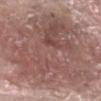  biopsy_status: not biopsied; imaged during a skin examination
  site: head or neck
  patient:
    sex: male
    age_approx: 65
  automated_metrics:
    eccentricity: 0.85
    shape_asymmetry: 0.2
    cielab_L: 53
    cielab_a: 19
    cielab_b: 22
    border_irregularity_0_10: 6.0
    nevus_likeness_0_100: 20
    lesion_detection_confidence_0_100: 95
  image:
    source: total-body photography crop
    field_of_view_mm: 15